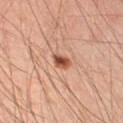This lesion was catalogued during total-body skin photography and was not selected for biopsy. A roughly 15 mm field-of-view crop from a total-body skin photograph. A male patient roughly 45 years of age. This is a cross-polarized tile. The lesion is located on the right upper arm.